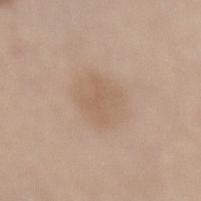| key | value |
|---|---|
| notes | no biopsy performed (imaged during a skin exam) |
| image source | ~15 mm tile from a whole-body skin photo |
| subject | male, roughly 65 years of age |
| image-analysis metrics | a within-lesion color-variation index near 2/10 and peripheral color asymmetry of about 0.5; an automated nevus-likeness rating near 5 out of 100 |
| body site | the back |
| lighting | white-light illumination |
| diameter | ~4.5 mm (longest diameter) |